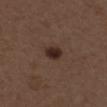Q: Was a biopsy performed?
A: total-body-photography surveillance lesion; no biopsy
Q: Patient demographics?
A: female, roughly 50 years of age
Q: Where on the body is the lesion?
A: the upper back
Q: What did automated image analysis measure?
A: a mean CIELAB color near L≈27 a*≈16 b*≈20, roughly 10 lightness units darker than nearby skin, and a normalized lesion–skin contrast near 10.5; border irregularity of about 2 on a 0–10 scale, a within-lesion color-variation index near 3/10, and peripheral color asymmetry of about 1
Q: What is the imaging modality?
A: ~15 mm tile from a whole-body skin photo
Q: How large is the lesion?
A: ~2.5 mm (longest diameter)